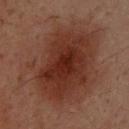Impression:
The lesion was photographed on a routine skin check and not biopsied; there is no pathology result.
Background:
Located on the upper back. About 11 mm across. The tile uses cross-polarized illumination. A 15 mm close-up tile from a total-body photography series done for melanoma screening. A male subject, roughly 50 years of age.